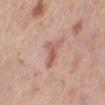<lesion>
<biopsy_status>not biopsied; imaged during a skin examination</biopsy_status>
<patient>
  <sex>female</sex>
  <age_approx>65</age_approx>
</patient>
<image>
  <source>total-body photography crop</source>
  <field_of_view_mm>15</field_of_view_mm>
</image>
<lesion_size>
  <long_diameter_mm_approx>3.5</long_diameter_mm_approx>
</lesion_size>
<automated_metrics>
  <area_mm2_approx>5.5</area_mm2_approx>
  <eccentricity>0.8</eccentricity>
  <shape_asymmetry>0.55</shape_asymmetry>
  <color_variation_0_10>4.0</color_variation_0_10>
  <peripheral_color_asymmetry>1.0</peripheral_color_asymmetry>
</automated_metrics>
<site>left lower leg</site>
</lesion>